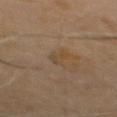From the back.
The tile uses cross-polarized illumination.
Longest diameter approximately 2.5 mm.
A lesion tile, about 15 mm wide, cut from a 3D total-body photograph.
Automated image analysis of the tile measured a mean CIELAB color near L≈43 a*≈13 b*≈30 and a lesion-to-skin contrast of about 4.5 (normalized; higher = more distinct).
A male subject aged 68–72.